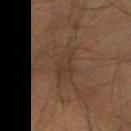Clinical impression: Captured during whole-body skin photography for melanoma surveillance; the lesion was not biopsied. Image and clinical context: About 4 mm across. A male subject, aged 43 to 47. Located on the right thigh. The tile uses cross-polarized illumination. A close-up tile cropped from a whole-body skin photograph, about 15 mm across.